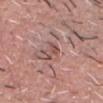Captured during whole-body skin photography for melanoma surveillance; the lesion was not biopsied. The tile uses white-light illumination. Cropped from a whole-body photographic skin survey; the tile spans about 15 mm. Automated tile analysis of the lesion measured a footprint of about 3.5 mm², an outline eccentricity of about 0.8 (0 = round, 1 = elongated), and two-axis asymmetry of about 0.3. The analysis additionally found a mean CIELAB color near L≈51 a*≈21 b*≈22, a lesion–skin lightness drop of about 9, and a lesion-to-skin contrast of about 6.5 (normalized; higher = more distinct). The software also gave a border-irregularity index near 4/10, internal color variation of about 1 on a 0–10 scale, and peripheral color asymmetry of about 0. The subject is a male aged approximately 60. The lesion is on the head or neck. Measured at roughly 2.5 mm in maximum diameter.